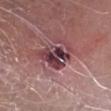Case summary:
* notes — catalogued during a skin exam; not biopsied
* location — the right lower leg
* patient — male, in their mid-60s
* automated metrics — a lesion area of about 12 mm², an outline eccentricity of about 0.8 (0 = round, 1 = elongated), and two-axis asymmetry of about 0.4; a lesion color around L≈40 a*≈25 b*≈15 in CIELAB, a lesion–skin lightness drop of about 14, and a normalized lesion–skin contrast near 11.5; border irregularity of about 5.5 on a 0–10 scale, internal color variation of about 8.5 on a 0–10 scale, and peripheral color asymmetry of about 2.5; an automated nevus-likeness rating near 0 out of 100 and a detector confidence of about 95 out of 100 that the crop contains a lesion
* imaging modality — total-body-photography crop, ~15 mm field of view
* tile lighting — white-light